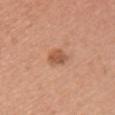Clinical impression: Captured during whole-body skin photography for melanoma surveillance; the lesion was not biopsied. Context: Captured under white-light illumination. A 15 mm close-up tile from a total-body photography series done for melanoma screening. Approximately 3 mm at its widest. The patient is a female aged around 40. The lesion is on the right upper arm.